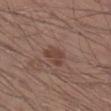Q: Is there a histopathology result?
A: catalogued during a skin exam; not biopsied
Q: What are the patient's age and sex?
A: male, in their 30s
Q: How was this image acquired?
A: 15 mm crop, total-body photography
Q: How large is the lesion?
A: about 3 mm
Q: What is the anatomic site?
A: the right lower leg
Q: What did automated image analysis measure?
A: an area of roughly 4.5 mm², an outline eccentricity of about 0.7 (0 = round, 1 = elongated), and a shape-asymmetry score of about 0.4 (0 = symmetric); an automated nevus-likeness rating near 10 out of 100 and a detector confidence of about 100 out of 100 that the crop contains a lesion
Q: Illumination type?
A: white-light illumination